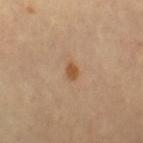* biopsy status · total-body-photography surveillance lesion; no biopsy
* diameter · ~2 mm (longest diameter)
* tile lighting · cross-polarized
* patient · female, roughly 60 years of age
* anatomic site · the left leg
* image · ~15 mm tile from a whole-body skin photo